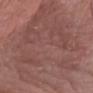Background:
A 15 mm crop from a total-body photograph taken for skin-cancer surveillance. The tile uses white-light illumination. The patient is a male in their mid-60s. Measured at roughly 17.5 mm in maximum diameter. Located on the chest.